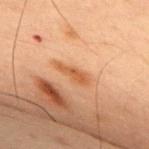Notes:
• notes — imaged on a skin check; not biopsied
• illumination — cross-polarized illumination
• diameter — ≈4.5 mm
• image source — ~15 mm tile from a whole-body skin photo
• patient — male, aged around 60
• anatomic site — the upper back